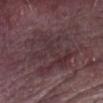{
  "biopsy_status": "not biopsied; imaged during a skin examination",
  "site": "front of the torso",
  "lesion_size": {
    "long_diameter_mm_approx": 9.0
  },
  "patient": {
    "sex": "male",
    "age_approx": 65
  },
  "automated_metrics": {
    "area_mm2_approx": 38.0,
    "eccentricity": 0.75,
    "cielab_L": 33,
    "cielab_a": 17,
    "cielab_b": 12,
    "vs_skin_darker_L": 6.0,
    "vs_skin_contrast_norm": 6.0,
    "border_irregularity_0_10": 5.0,
    "color_variation_0_10": 4.5,
    "peripheral_color_asymmetry": 1.5,
    "nevus_likeness_0_100": 0
  },
  "image": {
    "source": "total-body photography crop",
    "field_of_view_mm": 15
  },
  "lighting": "white-light"
}